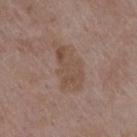Impression:
Captured during whole-body skin photography for melanoma surveillance; the lesion was not biopsied.
Acquisition and patient details:
On the upper back. A 15 mm close-up tile from a total-body photography series done for melanoma screening. A female patient, aged approximately 70.